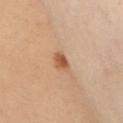Impression:
Imaged during a routine full-body skin examination; the lesion was not biopsied and no histopathology is available.
Context:
From the abdomen. An algorithmic analysis of the crop reported an area of roughly 3.5 mm² and an outline eccentricity of about 0.8 (0 = round, 1 = elongated). The software also gave a lesion color around L≈47 a*≈20 b*≈31 in CIELAB, a lesion–skin lightness drop of about 11, and a lesion-to-skin contrast of about 8.5 (normalized; higher = more distinct). And it measured border irregularity of about 2 on a 0–10 scale, a color-variation rating of about 2.5/10, and a peripheral color-asymmetry measure near 0.5. It also reported a nevus-likeness score of about 95/100. A female patient aged 58 to 62. This image is a 15 mm lesion crop taken from a total-body photograph. Longest diameter approximately 2.5 mm.